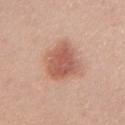biopsy_status: not biopsied; imaged during a skin examination
lesion_size:
  long_diameter_mm_approx: 5.0
image:
  source: total-body photography crop
  field_of_view_mm: 15
automated_metrics:
  area_mm2_approx: 14.0
  eccentricity: 0.75
  cielab_L: 57
  cielab_a: 25
  cielab_b: 29
  vs_skin_darker_L: 12.0
  vs_skin_contrast_norm: 8.0
  nevus_likeness_0_100: 100
  lesion_detection_confidence_0_100: 100
site: chest
patient:
  sex: female
  age_approx: 45
lighting: white-light